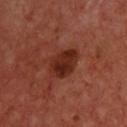<case>
  <biopsy_status>not biopsied; imaged during a skin examination</biopsy_status>
  <patient>
    <sex>male</sex>
    <age_approx>65</age_approx>
  </patient>
  <image>
    <source>total-body photography crop</source>
    <field_of_view_mm>15</field_of_view_mm>
  </image>
  <lighting>cross-polarized</lighting>
  <lesion_size>
    <long_diameter_mm_approx>4.0</long_diameter_mm_approx>
  </lesion_size>
  <site>back</site>
</case>